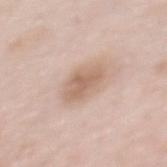{
  "biopsy_status": "not biopsied; imaged during a skin examination",
  "image": {
    "source": "total-body photography crop",
    "field_of_view_mm": 15
  },
  "patient": {
    "sex": "female",
    "age_approx": 65
  },
  "site": "mid back"
}